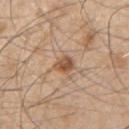Notes:
– TBP lesion metrics — a footprint of about 4.5 mm² and an eccentricity of roughly 0.7; an average lesion color of about L≈53 a*≈20 b*≈32 (CIELAB), about 12 CIELAB-L* units darker than the surrounding skin, and a lesion-to-skin contrast of about 8 (normalized; higher = more distinct); a color-variation rating of about 4/10 and peripheral color asymmetry of about 1.5
– site — the right upper arm
– subject — male, aged 48–52
– diameter — ≈3 mm
– image — ~15 mm crop, total-body skin-cancer survey